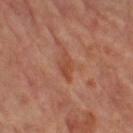No biopsy was performed on this lesion — it was imaged during a full skin examination and was not determined to be concerning. A 15 mm crop from a total-body photograph taken for skin-cancer surveillance. The tile uses cross-polarized illumination. From the left leg. The subject is a female in their mid-60s. Automated tile analysis of the lesion measured a lesion area of about 4 mm² and a shape eccentricity near 0.9. The analysis additionally found a nevus-likeness score of about 0/100.